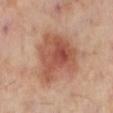biopsy status — imaged on a skin check; not biopsied
automated metrics — a classifier nevus-likeness of about 100/100 and lesion-presence confidence of about 100/100
site — the left lower leg
lesion diameter — ~7 mm (longest diameter)
lighting — cross-polarized illumination
acquisition — 15 mm crop, total-body photography
subject — female, in their mid- to late 50s